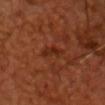The lesion was photographed on a routine skin check and not biopsied; there is no pathology result. From the head or neck. This image is a 15 mm lesion crop taken from a total-body photograph. About 3.5 mm across. A male subject, roughly 50 years of age.